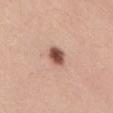{
  "site": "back",
  "patient": {
    "sex": "male",
    "age_approx": 55
  },
  "lighting": "white-light",
  "image": {
    "source": "total-body photography crop",
    "field_of_view_mm": 15
  },
  "automated_metrics": {
    "border_irregularity_0_10": 1.5,
    "color_variation_0_10": 4.0,
    "peripheral_color_asymmetry": 1.0
  }
}